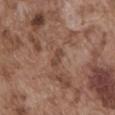* biopsy status: total-body-photography surveillance lesion; no biopsy
* tile lighting: white-light illumination
* diameter: ≈2.5 mm
* image source: ~15 mm crop, total-body skin-cancer survey
* patient: male, in their mid-70s
* TBP lesion metrics: a lesion area of about 2.5 mm² and an eccentricity of roughly 0.9; a lesion color around L≈44 a*≈20 b*≈26 in CIELAB, a lesion–skin lightness drop of about 8, and a normalized lesion–skin contrast near 6.5
* body site: the abdomen